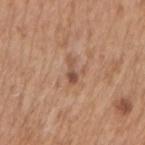This lesion was catalogued during total-body skin photography and was not selected for biopsy.
A male patient in their 60s.
The lesion's longest dimension is about 3 mm.
Captured under white-light illumination.
From the right upper arm.
A 15 mm close-up extracted from a 3D total-body photography capture.